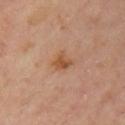Clinical impression: This lesion was catalogued during total-body skin photography and was not selected for biopsy. Image and clinical context: A 15 mm close-up extracted from a 3D total-body photography capture. The lesion is located on the left upper arm. The patient is a female aged 43–47.